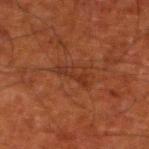Impression:
Recorded during total-body skin imaging; not selected for excision or biopsy.
Context:
The lesion is located on the left lower leg. Imaged with cross-polarized lighting. The patient is a male roughly 80 years of age. A 15 mm close-up extracted from a 3D total-body photography capture. The lesion's longest dimension is about 4 mm.